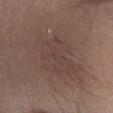Q: Is there a histopathology result?
A: total-body-photography surveillance lesion; no biopsy
Q: Who is the patient?
A: male, roughly 55 years of age
Q: Lesion size?
A: ~8 mm (longest diameter)
Q: What did automated image analysis measure?
A: border irregularity of about 3.5 on a 0–10 scale, a within-lesion color-variation index near 2/10, and peripheral color asymmetry of about 0.5; a nevus-likeness score of about 0/100
Q: How was the tile lit?
A: white-light
Q: What kind of image is this?
A: ~15 mm tile from a whole-body skin photo
Q: Where on the body is the lesion?
A: the right lower leg